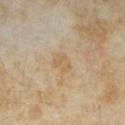Imaged during a routine full-body skin examination; the lesion was not biopsied and no histopathology is available. An algorithmic analysis of the crop reported roughly 5 lightness units darker than nearby skin and a lesion-to-skin contrast of about 5 (normalized; higher = more distinct). A female subject aged 33–37. A 15 mm close-up extracted from a 3D total-body photography capture. On the right forearm. The tile uses cross-polarized illumination. Measured at roughly 2.5 mm in maximum diameter.